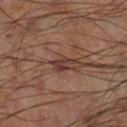Clinical impression:
This lesion was catalogued during total-body skin photography and was not selected for biopsy.
Clinical summary:
A close-up tile cropped from a whole-body skin photograph, about 15 mm across. The tile uses cross-polarized illumination. The lesion's longest dimension is about 3 mm. The lesion is located on the right lower leg.